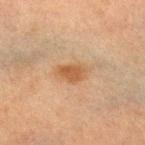Q: Automated lesion metrics?
A: a shape eccentricity near 0.8 and two-axis asymmetry of about 0.3; a normalized lesion–skin contrast near 7.5; a nevus-likeness score of about 90/100 and a detector confidence of about 100 out of 100 that the crop contains a lesion
Q: What is the imaging modality?
A: 15 mm crop, total-body photography
Q: What are the patient's age and sex?
A: female, aged around 55
Q: How large is the lesion?
A: about 3.5 mm
Q: Where on the body is the lesion?
A: the right lower leg
Q: Illumination type?
A: cross-polarized